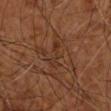Imaged during a routine full-body skin examination; the lesion was not biopsied and no histopathology is available. Cropped from a total-body skin-imaging series; the visible field is about 15 mm. The lesion's longest dimension is about 3.5 mm. The patient is aged approximately 65. From the right upper arm.